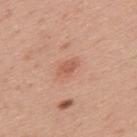This lesion was catalogued during total-body skin photography and was not selected for biopsy. The lesion is on the back. A male subject, about 30 years old. This image is a 15 mm lesion crop taken from a total-body photograph. About 2.5 mm across.